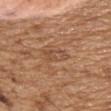biopsy status: total-body-photography surveillance lesion; no biopsy
patient: male, aged around 70
site: the head or neck
TBP lesion metrics: border irregularity of about 3 on a 0–10 scale, internal color variation of about 3.5 on a 0–10 scale, and a peripheral color-asymmetry measure near 1.5; lesion-presence confidence of about 85/100
acquisition: 15 mm crop, total-body photography
diameter: ~3.5 mm (longest diameter)